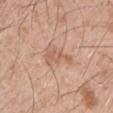follow-up — total-body-photography surveillance lesion; no biopsy | lesion size — about 4 mm | site — the left upper arm | tile lighting — white-light illumination | imaging modality — 15 mm crop, total-body photography | patient — male, in their 50s | automated lesion analysis — a lesion area of about 5.5 mm², a shape eccentricity near 0.85, and two-axis asymmetry of about 0.55; an average lesion color of about L≈60 a*≈21 b*≈30 (CIELAB), about 8 CIELAB-L* units darker than the surrounding skin, and a lesion-to-skin contrast of about 5.5 (normalized; higher = more distinct); a nevus-likeness score of about 0/100 and a lesion-detection confidence of about 100/100.